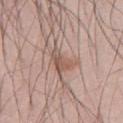<lesion>
<biopsy_status>not biopsied; imaged during a skin examination</biopsy_status>
<lighting>white-light</lighting>
<site>abdomen</site>
<lesion_size>
  <long_diameter_mm_approx>3.5</long_diameter_mm_approx>
</lesion_size>
<patient>
  <sex>male</sex>
  <age_approx>55</age_approx>
</patient>
<image>
  <source>total-body photography crop</source>
  <field_of_view_mm>15</field_of_view_mm>
</image>
</lesion>